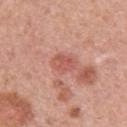Context: From the left upper arm. The tile uses white-light illumination. The patient is a female roughly 60 years of age. A lesion tile, about 15 mm wide, cut from a 3D total-body photograph.